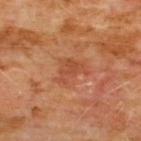Impression:
No biopsy was performed on this lesion — it was imaged during a full skin examination and was not determined to be concerning.
Image and clinical context:
Located on the chest. About 3.5 mm across. A male patient roughly 60 years of age. A 15 mm close-up tile from a total-body photography series done for melanoma screening. The lesion-visualizer software estimated a mean CIELAB color near L≈47 a*≈27 b*≈35 and about 7 CIELAB-L* units darker than the surrounding skin. Imaged with cross-polarized lighting.